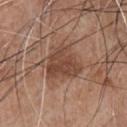Q: Was this lesion biopsied?
A: no biopsy performed (imaged during a skin exam)
Q: What kind of image is this?
A: ~15 mm tile from a whole-body skin photo
Q: Who is the patient?
A: male, aged 63 to 67
Q: How was the tile lit?
A: white-light illumination
Q: Where on the body is the lesion?
A: the chest
Q: Lesion size?
A: ~4.5 mm (longest diameter)